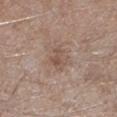workup: total-body-photography surveillance lesion; no biopsy | TBP lesion metrics: an eccentricity of roughly 0.65 and two-axis asymmetry of about 0.3; an average lesion color of about L≈52 a*≈16 b*≈24 (CIELAB), roughly 7 lightness units darker than nearby skin, and a lesion-to-skin contrast of about 5.5 (normalized; higher = more distinct); a classifier nevus-likeness of about 0/100 | lesion diameter: ~3 mm (longest diameter) | patient: male, roughly 65 years of age | body site: the left lower leg | acquisition: total-body-photography crop, ~15 mm field of view | lighting: white-light.